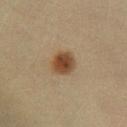{"biopsy_status": "not biopsied; imaged during a skin examination", "site": "left lower leg", "lighting": "cross-polarized", "image": {"source": "total-body photography crop", "field_of_view_mm": 15}, "patient": {"sex": "female", "age_approx": 40}}